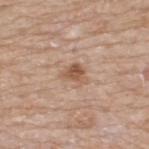| field | value |
|---|---|
| biopsy status | no biopsy performed (imaged during a skin exam) |
| location | the upper back |
| image source | ~15 mm tile from a whole-body skin photo |
| image-analysis metrics | a lesion area of about 5 mm², an eccentricity of roughly 0.7, and a shape-asymmetry score of about 0.4 (0 = symmetric); a lesion–skin lightness drop of about 10 and a normalized lesion–skin contrast near 7.5 |
| patient | male, aged approximately 65 |